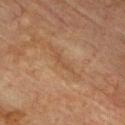This lesion was catalogued during total-body skin photography and was not selected for biopsy. A 15 mm close-up extracted from a 3D total-body photography capture. A male patient, aged approximately 75. This is a cross-polarized tile. Located on the chest. The recorded lesion diameter is about 3.5 mm.